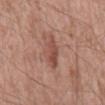notes = imaged on a skin check; not biopsied
lesion diameter = about 4 mm
anatomic site = the mid back
subject = male, approximately 60 years of age
image = ~15 mm tile from a whole-body skin photo
illumination = white-light illumination